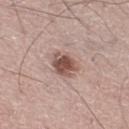biopsy status = catalogued during a skin exam; not biopsied | subject = male, about 55 years old | size = ≈3 mm | illumination = white-light illumination | image source = total-body-photography crop, ~15 mm field of view | TBP lesion metrics = a border-irregularity index near 2.5/10, a within-lesion color-variation index near 4.5/10, and radial color variation of about 1.5.